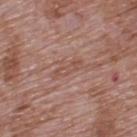Part of a total-body skin-imaging series; this lesion was reviewed on a skin check and was not flagged for biopsy.
Located on the upper back.
Longest diameter approximately 3 mm.
A male patient, aged around 70.
This image is a 15 mm lesion crop taken from a total-body photograph.
The tile uses white-light illumination.